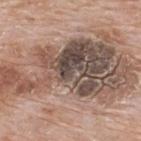The lesion was tiled from a total-body skin photograph and was not biopsied.